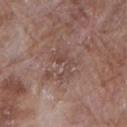Imaged during a routine full-body skin examination; the lesion was not biopsied and no histopathology is available. The lesion-visualizer software estimated a border-irregularity index near 9/10, internal color variation of about 2 on a 0–10 scale, and peripheral color asymmetry of about 0.5. The software also gave a lesion-detection confidence of about 95/100. A lesion tile, about 15 mm wide, cut from a 3D total-body photograph. A female subject, in their 70s. The lesion is located on the right upper arm.